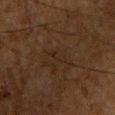follow-up: total-body-photography surveillance lesion; no biopsy | image source: ~15 mm crop, total-body skin-cancer survey | lesion size: ~3 mm (longest diameter) | body site: the chest | subject: male, aged approximately 65 | tile lighting: cross-polarized illumination | automated lesion analysis: a footprint of about 2 mm², an eccentricity of roughly 0.95, and a shape-asymmetry score of about 0.65 (0 = symmetric); an average lesion color of about L≈19 a*≈12 b*≈20 (CIELAB), a lesion–skin lightness drop of about 3, and a lesion-to-skin contrast of about 4.5 (normalized; higher = more distinct); lesion-presence confidence of about 75/100.